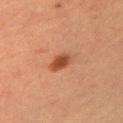Q: Is there a histopathology result?
A: no biopsy performed (imaged during a skin exam)
Q: Patient demographics?
A: female, roughly 55 years of age
Q: What is the anatomic site?
A: the left upper arm
Q: What kind of image is this?
A: ~15 mm crop, total-body skin-cancer survey